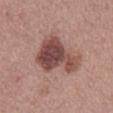Assessment:
This lesion was catalogued during total-body skin photography and was not selected for biopsy.
Acquisition and patient details:
The subject is a male approximately 55 years of age. About 6.5 mm across. Imaged with white-light lighting. On the mid back. A 15 mm close-up tile from a total-body photography series done for melanoma screening.